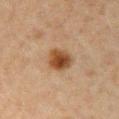{"biopsy_status": "not biopsied; imaged during a skin examination", "image": {"source": "total-body photography crop", "field_of_view_mm": 15}, "patient": {"sex": "female", "age_approx": 40}, "lesion_size": {"long_diameter_mm_approx": 3.0}, "lighting": "cross-polarized", "site": "chest"}